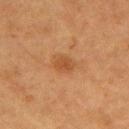Q: How large is the lesion?
A: about 3 mm
Q: Illumination type?
A: cross-polarized
Q: Automated lesion metrics?
A: a lesion color around L≈41 a*≈21 b*≈33 in CIELAB, roughly 7 lightness units darker than nearby skin, and a lesion-to-skin contrast of about 6 (normalized; higher = more distinct)
Q: Patient demographics?
A: female, aged around 55
Q: How was this image acquired?
A: ~15 mm tile from a whole-body skin photo
Q: Where on the body is the lesion?
A: the left upper arm